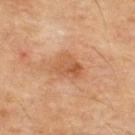Impression:
Imaged during a routine full-body skin examination; the lesion was not biopsied and no histopathology is available.
Background:
Cropped from a whole-body photographic skin survey; the tile spans about 15 mm. Captured under cross-polarized illumination. A male patient in their mid- to late 50s. The lesion is located on the upper back. Measured at roughly 4 mm in maximum diameter.